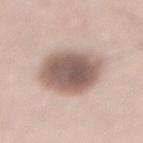<lesion>
<biopsy_status>not biopsied; imaged during a skin examination</biopsy_status>
<patient>
  <sex>male</sex>
  <age_approx>65</age_approx>
</patient>
<image>
  <source>total-body photography crop</source>
  <field_of_view_mm>15</field_of_view_mm>
</image>
<lesion_size>
  <long_diameter_mm_approx>6.5</long_diameter_mm_approx>
</lesion_size>
<site>lower back</site>
</lesion>